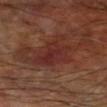No biopsy was performed on this lesion — it was imaged during a full skin examination and was not determined to be concerning.
A male subject aged 58 to 62.
The lesion is on the leg.
Imaged with cross-polarized lighting.
Approximately 5.5 mm at its widest.
Automated tile analysis of the lesion measured a nevus-likeness score of about 0/100.
A lesion tile, about 15 mm wide, cut from a 3D total-body photograph.